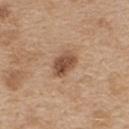Assessment: No biopsy was performed on this lesion — it was imaged during a full skin examination and was not determined to be concerning. Image and clinical context: A female patient about 45 years old. About 3.5 mm across. An algorithmic analysis of the crop reported a lesion color around L≈50 a*≈20 b*≈31 in CIELAB, a lesion–skin lightness drop of about 13, and a lesion-to-skin contrast of about 9 (normalized; higher = more distinct). The analysis additionally found a lesion-detection confidence of about 100/100. Located on the upper back. A lesion tile, about 15 mm wide, cut from a 3D total-body photograph.